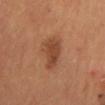Q: Was a biopsy performed?
A: total-body-photography surveillance lesion; no biopsy
Q: Where on the body is the lesion?
A: the mid back
Q: Automated lesion metrics?
A: a within-lesion color-variation index near 2.5/10 and peripheral color asymmetry of about 0.5; a nevus-likeness score of about 65/100 and lesion-presence confidence of about 100/100
Q: What lighting was used for the tile?
A: cross-polarized illumination
Q: What kind of image is this?
A: total-body-photography crop, ~15 mm field of view
Q: What is the lesion's diameter?
A: ≈5 mm
Q: Patient demographics?
A: male, in their mid- to late 50s